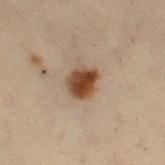– biopsy status · imaged on a skin check; not biopsied
– subject · female, aged 48–52
– acquisition · total-body-photography crop, ~15 mm field of view
– site · the right thigh